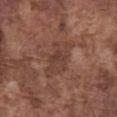  biopsy_status: not biopsied; imaged during a skin examination
  site: chest
  image:
    source: total-body photography crop
    field_of_view_mm: 15
  patient:
    sex: male
    age_approx: 75